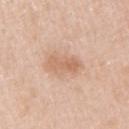Notes:
– notes · catalogued during a skin exam; not biopsied
– size · about 3.5 mm
– image-analysis metrics · an area of roughly 5 mm² and a symmetry-axis asymmetry near 0.25; internal color variation of about 3 on a 0–10 scale and peripheral color asymmetry of about 1; a nevus-likeness score of about 10/100 and a detector confidence of about 100 out of 100 that the crop contains a lesion
– subject · female, aged around 45
– acquisition · 15 mm crop, total-body photography
– anatomic site · the arm